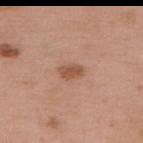| field | value |
|---|---|
| biopsy status | total-body-photography surveillance lesion; no biopsy |
| subject | female, aged 68–72 |
| image-analysis metrics | a lesion area of about 3.5 mm², an outline eccentricity of about 0.85 (0 = round, 1 = elongated), and a shape-asymmetry score of about 0.25 (0 = symmetric); an automated nevus-likeness rating near 80 out of 100 and a lesion-detection confidence of about 100/100 |
| image source | ~15 mm crop, total-body skin-cancer survey |
| diameter | about 2.5 mm |
| site | the upper back |
| tile lighting | white-light illumination |